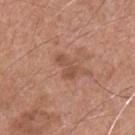follow-up: imaged on a skin check; not biopsied | illumination: white-light illumination | acquisition: total-body-photography crop, ~15 mm field of view | patient: male, about 55 years old | automated metrics: a lesion area of about 4.5 mm² and an outline eccentricity of about 0.85 (0 = round, 1 = elongated); a lesion color around L≈51 a*≈23 b*≈30 in CIELAB and a normalized border contrast of about 6; lesion-presence confidence of about 100/100 | anatomic site: the front of the torso.